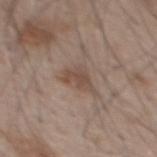Q: Was this lesion biopsied?
A: no biopsy performed (imaged during a skin exam)
Q: Illumination type?
A: white-light illumination
Q: What is the anatomic site?
A: the mid back
Q: What is the lesion's diameter?
A: ≈3.5 mm
Q: Patient demographics?
A: male, roughly 50 years of age
Q: What is the imaging modality?
A: ~15 mm crop, total-body skin-cancer survey